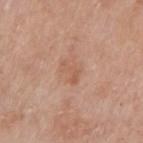Recorded during total-body skin imaging; not selected for excision or biopsy.